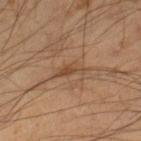Imaged during a routine full-body skin examination; the lesion was not biopsied and no histopathology is available.
Cropped from a total-body skin-imaging series; the visible field is about 15 mm.
The lesion is located on the right lower leg.
The lesion's longest dimension is about 3.5 mm.
Imaged with cross-polarized lighting.
A male subject roughly 40 years of age.